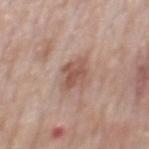biopsy status — catalogued during a skin exam; not biopsied | image source — 15 mm crop, total-body photography | tile lighting — white-light illumination | lesion diameter — about 3.5 mm | patient — male, aged approximately 75 | location — the mid back.